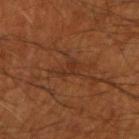The lesion was tiled from a total-body skin photograph and was not biopsied. The lesion is located on the right forearm. Automated tile analysis of the lesion measured a footprint of about 4 mm², an eccentricity of roughly 0.8, and a shape-asymmetry score of about 0.5 (0 = symmetric). The software also gave an average lesion color of about L≈31 a*≈22 b*≈30 (CIELAB) and a normalized border contrast of about 5.5. A 15 mm crop from a total-body photograph taken for skin-cancer surveillance. Longest diameter approximately 3 mm. The patient is a male aged 53 to 57.